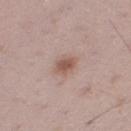The lesion was tiled from a total-body skin photograph and was not biopsied. A male patient, about 50 years old. The lesion is on the right thigh. This image is a 15 mm lesion crop taken from a total-body photograph.